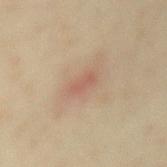Clinical impression: Recorded during total-body skin imaging; not selected for excision or biopsy. Image and clinical context: A female patient roughly 35 years of age. Longest diameter approximately 2.5 mm. The lesion-visualizer software estimated a lesion area of about 2 mm². The analysis additionally found border irregularity of about 4.5 on a 0–10 scale and radial color variation of about 0. The analysis additionally found a lesion-detection confidence of about 100/100. The lesion is located on the mid back. A 15 mm close-up extracted from a 3D total-body photography capture. This is a cross-polarized tile.